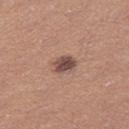Notes:
- image source — total-body-photography crop, ~15 mm field of view
- automated lesion analysis — an eccentricity of roughly 0.6 and two-axis asymmetry of about 0.2; a mean CIELAB color near L≈47 a*≈19 b*≈22, a lesion–skin lightness drop of about 14, and a normalized lesion–skin contrast near 10.5; a border-irregularity index near 2/10, a within-lesion color-variation index near 3/10, and radial color variation of about 1; a nevus-likeness score of about 50/100 and lesion-presence confidence of about 100/100
- subject — female, aged 38 to 42
- lighting — white-light
- body site — the right thigh
- size — ≈2.5 mm